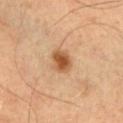Assessment:
No biopsy was performed on this lesion — it was imaged during a full skin examination and was not determined to be concerning.
Clinical summary:
A male patient, aged 53–57. The lesion's longest dimension is about 3 mm. The lesion is located on the chest. A 15 mm crop from a total-body photograph taken for skin-cancer surveillance. This is a cross-polarized tile.